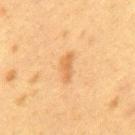  biopsy_status: not biopsied; imaged during a skin examination
  patient:
    sex: female
    age_approx: 40
  site: mid back
  image:
    source: total-body photography crop
    field_of_view_mm: 15
  lighting: cross-polarized
  lesion_size:
    long_diameter_mm_approx: 3.5